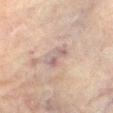Impression:
The lesion was photographed on a routine skin check and not biopsied; there is no pathology result.
Clinical summary:
A lesion tile, about 15 mm wide, cut from a 3D total-body photograph. Measured at roughly 3 mm in maximum diameter. Automated image analysis of the tile measured a lesion color around L≈54 a*≈14 b*≈19 in CIELAB, about 7 CIELAB-L* units darker than the surrounding skin, and a normalized lesion–skin contrast near 5.5. It also reported a border-irregularity rating of about 4/10, a color-variation rating of about 5.5/10, and peripheral color asymmetry of about 2. The subject is a female aged 78 to 82. Captured under cross-polarized illumination. The lesion is located on the right thigh.